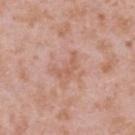{
  "biopsy_status": "not biopsied; imaged during a skin examination",
  "patient": {
    "sex": "female",
    "age_approx": 40
  },
  "site": "upper back",
  "image": {
    "source": "total-body photography crop",
    "field_of_view_mm": 15
  }
}